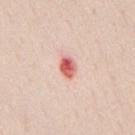• follow-up — no biopsy performed (imaged during a skin exam)
• body site — the mid back
• subject — male, aged 33–37
• image — 15 mm crop, total-body photography
• illumination — white-light
• automated metrics — a footprint of about 4.5 mm², a shape eccentricity near 0.75, and a shape-asymmetry score of about 0.1 (0 = symmetric); roughly 16 lightness units darker than nearby skin and a lesion-to-skin contrast of about 9.5 (normalized; higher = more distinct); a classifier nevus-likeness of about 0/100 and a lesion-detection confidence of about 100/100